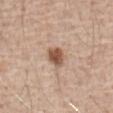No biopsy was performed on this lesion — it was imaged during a full skin examination and was not determined to be concerning. From the abdomen. Imaged with white-light lighting. Cropped from a total-body skin-imaging series; the visible field is about 15 mm. The subject is a male in their mid- to late 60s. Approximately 3 mm at its widest.